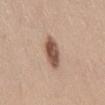The lesion was tiled from a total-body skin photograph and was not biopsied. This image is a 15 mm lesion crop taken from a total-body photograph. The subject is a male aged approximately 55. The lesion is on the abdomen. The total-body-photography lesion software estimated a normalized border contrast of about 10.5. Longest diameter approximately 4 mm.